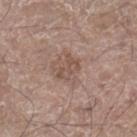{
  "biopsy_status": "not biopsied; imaged during a skin examination",
  "lighting": "white-light",
  "image": {
    "source": "total-body photography crop",
    "field_of_view_mm": 15
  },
  "lesion_size": {
    "long_diameter_mm_approx": 3.0
  },
  "automated_metrics": {
    "lesion_detection_confidence_0_100": 100
  },
  "site": "right lower leg",
  "patient": {
    "sex": "male",
    "age_approx": 60
  }
}